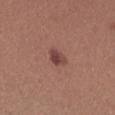Background:
The patient is a female aged around 30. Automated tile analysis of the lesion measured a lesion–skin lightness drop of about 11 and a lesion-to-skin contrast of about 8.5 (normalized; higher = more distinct). The software also gave a border-irregularity rating of about 2.5/10, a within-lesion color-variation index near 2.5/10, and peripheral color asymmetry of about 1. The analysis additionally found a classifier nevus-likeness of about 90/100. This is a white-light tile. A close-up tile cropped from a whole-body skin photograph, about 15 mm across. Longest diameter approximately 2.5 mm. The lesion is on the right upper arm.